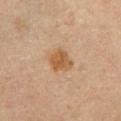Part of a total-body skin-imaging series; this lesion was reviewed on a skin check and was not flagged for biopsy. On the abdomen. This is a cross-polarized tile. The recorded lesion diameter is about 3 mm. Cropped from a whole-body photographic skin survey; the tile spans about 15 mm. Automated tile analysis of the lesion measured a footprint of about 7 mm², an outline eccentricity of about 0.55 (0 = round, 1 = elongated), and a symmetry-axis asymmetry near 0.25. The software also gave a border-irregularity index near 2.5/10, a within-lesion color-variation index near 3/10, and radial color variation of about 1. A male patient, in their mid- to late 60s.